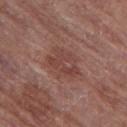No biopsy was performed on this lesion — it was imaged during a full skin examination and was not determined to be concerning.
A 15 mm crop from a total-body photograph taken for skin-cancer surveillance.
The recorded lesion diameter is about 4.5 mm.
The lesion is located on the left thigh.
Imaged with white-light lighting.
A female subject, aged approximately 80.